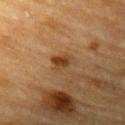notes: catalogued during a skin exam; not biopsied | subject: male, approximately 85 years of age or older | location: the left upper arm | tile lighting: cross-polarized | lesion diameter: ≈2.5 mm | imaging modality: total-body-photography crop, ~15 mm field of view.